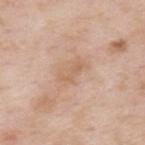{"biopsy_status": "not biopsied; imaged during a skin examination", "site": "back", "patient": {"sex": "male", "age_approx": 55}, "image": {"source": "total-body photography crop", "field_of_view_mm": 15}}